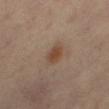<case>
  <biopsy_status>not biopsied; imaged during a skin examination</biopsy_status>
  <site>right thigh</site>
  <lesion_size>
    <long_diameter_mm_approx>3.5</long_diameter_mm_approx>
  </lesion_size>
  <image>
    <source>total-body photography crop</source>
    <field_of_view_mm>15</field_of_view_mm>
  </image>
  <patient>
    <sex>male</sex>
    <age_approx>60</age_approx>
  </patient>
  <lighting>cross-polarized</lighting>
  <automated_metrics>
    <eccentricity>0.8</eccentricity>
    <shape_asymmetry>0.2</shape_asymmetry>
    <border_irregularity_0_10>2.0</border_irregularity_0_10>
    <color_variation_0_10>3.0</color_variation_0_10>
    <peripheral_color_asymmetry>1.0</peripheral_color_asymmetry>
  </automated_metrics>
</case>